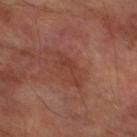Part of a total-body skin-imaging series; this lesion was reviewed on a skin check and was not flagged for biopsy. The total-body-photography lesion software estimated a lesion color around L≈39 a*≈24 b*≈27 in CIELAB, roughly 6 lightness units darker than nearby skin, and a normalized border contrast of about 5. A male subject aged 68–72. Captured under cross-polarized illumination. The lesion is on the left lower leg. A lesion tile, about 15 mm wide, cut from a 3D total-body photograph. Longest diameter approximately 4.5 mm.